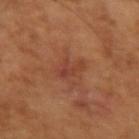notes: total-body-photography surveillance lesion; no biopsy
imaging modality: 15 mm crop, total-body photography
anatomic site: the left upper arm
subject: male, aged approximately 65